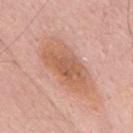<case>
  <biopsy_status>not biopsied; imaged during a skin examination</biopsy_status>
  <lesion_size>
    <long_diameter_mm_approx>7.5</long_diameter_mm_approx>
  </lesion_size>
  <lighting>white-light</lighting>
  <image>
    <source>total-body photography crop</source>
    <field_of_view_mm>15</field_of_view_mm>
  </image>
  <automated_metrics>
    <area_mm2_approx>24.0</area_mm2_approx>
    <eccentricity>0.9</eccentricity>
    <shape_asymmetry>0.2</shape_asymmetry>
    <cielab_L>61</cielab_L>
    <cielab_a>23</cielab_a>
    <cielab_b>32</cielab_b>
    <vs_skin_darker_L>9.0</vs_skin_darker_L>
    <vs_skin_contrast_norm>7.0</vs_skin_contrast_norm>
  </automated_metrics>
  <site>mid back</site>
  <patient>
    <sex>male</sex>
    <age_approx>65</age_approx>
  </patient>
</case>